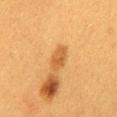| feature | finding |
|---|---|
| follow-up | imaged on a skin check; not biopsied |
| imaging modality | ~15 mm crop, total-body skin-cancer survey |
| anatomic site | the mid back |
| subject | female, aged approximately 40 |
| diameter | ≈3.5 mm |